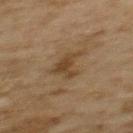This lesion was catalogued during total-body skin photography and was not selected for biopsy. Imaged with cross-polarized lighting. The lesion is on the upper back. Approximately 3.5 mm at its widest. The subject is a female approximately 60 years of age. A 15 mm close-up tile from a total-body photography series done for melanoma screening.